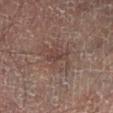Context: Automated image analysis of the tile measured an average lesion color of about L≈36 a*≈16 b*≈19 (CIELAB), a lesion–skin lightness drop of about 6, and a normalized lesion–skin contrast near 5.5. The recorded lesion diameter is about 3 mm. Located on the left lower leg. A roughly 15 mm field-of-view crop from a total-body skin photograph. A male subject, aged around 50. This is a cross-polarized tile.